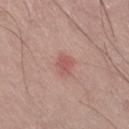Imaged during a routine full-body skin examination; the lesion was not biopsied and no histopathology is available.
On the right thigh.
Cropped from a whole-body photographic skin survey; the tile spans about 15 mm.
A male subject, approximately 55 years of age.